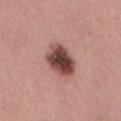Q: Is there a histopathology result?
A: no biopsy performed (imaged during a skin exam)
Q: What kind of image is this?
A: 15 mm crop, total-body photography
Q: How was the tile lit?
A: white-light illumination
Q: Lesion location?
A: the right thigh
Q: Lesion size?
A: ~4.5 mm (longest diameter)
Q: Patient demographics?
A: female, approximately 30 years of age